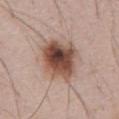The lesion was photographed on a routine skin check and not biopsied; there is no pathology result. The patient is a male aged approximately 55. Longest diameter approximately 5.5 mm. From the abdomen. Cropped from a total-body skin-imaging series; the visible field is about 15 mm. This is a white-light tile.